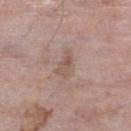{
  "biopsy_status": "not biopsied; imaged during a skin examination",
  "image": {
    "source": "total-body photography crop",
    "field_of_view_mm": 15
  },
  "site": "leg",
  "patient": {
    "sex": "male",
    "age_approx": 75
  },
  "automated_metrics": {
    "area_mm2_approx": 4.0,
    "eccentricity": 0.85,
    "shape_asymmetry": 0.4,
    "cielab_L": 54,
    "cielab_a": 17,
    "cielab_b": 25,
    "vs_skin_darker_L": 7.0
  }
}